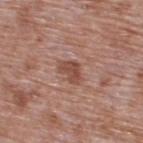A male subject, aged approximately 70.
This is a white-light tile.
The total-body-photography lesion software estimated an automated nevus-likeness rating near 50 out of 100 and lesion-presence confidence of about 100/100.
A region of skin cropped from a whole-body photographic capture, roughly 15 mm wide.
From the upper back.
The lesion's longest dimension is about 3 mm.